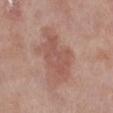- biopsy status — no biopsy performed (imaged during a skin exam)
- illumination — white-light
- lesion size — ≈6.5 mm
- subject — female, approximately 70 years of age
- automated lesion analysis — a detector confidence of about 100 out of 100 that the crop contains a lesion
- image — ~15 mm tile from a whole-body skin photo
- site — the leg